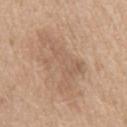{
  "biopsy_status": "not biopsied; imaged during a skin examination",
  "patient": {
    "sex": "male",
    "age_approx": 70
  },
  "lighting": "white-light",
  "image": {
    "source": "total-body photography crop",
    "field_of_view_mm": 15
  },
  "site": "front of the torso",
  "lesion_size": {
    "long_diameter_mm_approx": 7.5
  }
}